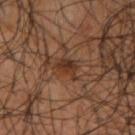Findings:
- biopsy status: catalogued during a skin exam; not biopsied
- tile lighting: cross-polarized
- diameter: ~3 mm (longest diameter)
- image source: total-body-photography crop, ~15 mm field of view
- patient: male, aged approximately 40
- location: the right upper arm